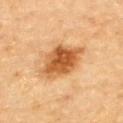Q: Was this lesion biopsied?
A: total-body-photography surveillance lesion; no biopsy
Q: Where on the body is the lesion?
A: the back
Q: What kind of image is this?
A: 15 mm crop, total-body photography
Q: How large is the lesion?
A: ~5.5 mm (longest diameter)
Q: What did automated image analysis measure?
A: a lesion area of about 16 mm², an outline eccentricity of about 0.7 (0 = round, 1 = elongated), and a symmetry-axis asymmetry near 0.2; a lesion color around L≈57 a*≈25 b*≈43 in CIELAB and a lesion–skin lightness drop of about 15; border irregularity of about 2 on a 0–10 scale, a color-variation rating of about 6/10, and radial color variation of about 2; an automated nevus-likeness rating near 95 out of 100 and a detector confidence of about 100 out of 100 that the crop contains a lesion
Q: What are the patient's age and sex?
A: male, aged 83 or over
Q: How was the tile lit?
A: cross-polarized illumination